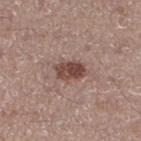The lesion is located on the right thigh. A 15 mm crop from a total-body photograph taken for skin-cancer surveillance. The recorded lesion diameter is about 3.5 mm. The subject is a female in their 50s. Imaged with white-light lighting.